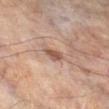{"biopsy_status": "not biopsied; imaged during a skin examination", "image": {"source": "total-body photography crop", "field_of_view_mm": 15}, "lighting": "cross-polarized", "site": "right lower leg", "lesion_size": {"long_diameter_mm_approx": 2.5}, "automated_metrics": {"area_mm2_approx": 3.5, "eccentricity": 0.8, "shape_asymmetry": 0.25}, "patient": {"sex": "male", "age_approx": 65}}